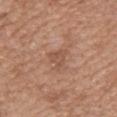Assessment: Captured during whole-body skin photography for melanoma surveillance; the lesion was not biopsied. Context: The lesion is located on the upper back. The patient is a female in their 40s. Measured at roughly 2.5 mm in maximum diameter. A roughly 15 mm field-of-view crop from a total-body skin photograph. Imaged with white-light lighting.